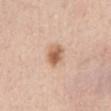The lesion was photographed on a routine skin check and not biopsied; there is no pathology result. The patient is a female in their 40s. A close-up tile cropped from a whole-body skin photograph, about 15 mm across. Captured under white-light illumination. Located on the chest.